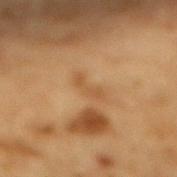No biopsy was performed on this lesion — it was imaged during a full skin examination and was not determined to be concerning. Approximately 3.5 mm at its widest. The lesion-visualizer software estimated an eccentricity of roughly 0.95. And it measured a lesion color around L≈46 a*≈17 b*≈34 in CIELAB and a normalized border contrast of about 5. The lesion is located on the mid back. A 15 mm crop from a total-body photograph taken for skin-cancer surveillance. The patient is a male in their mid-80s or older.